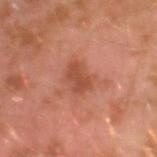automated metrics = a lesion color around L≈48 a*≈27 b*≈32 in CIELAB, about 9 CIELAB-L* units darker than the surrounding skin, and a normalized border contrast of about 6.5; a border-irregularity rating of about 2.5/10 and radial color variation of about 0.5; an automated nevus-likeness rating near 5 out of 100 and lesion-presence confidence of about 100/100
patient = male, roughly 30 years of age
location = the arm
size = ≈3.5 mm
image = ~15 mm crop, total-body skin-cancer survey